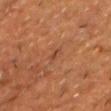{
  "biopsy_status": "not biopsied; imaged during a skin examination",
  "lesion_size": {
    "long_diameter_mm_approx": 2.5
  },
  "image": {
    "source": "total-body photography crop",
    "field_of_view_mm": 15
  },
  "site": "chest",
  "automated_metrics": {
    "lesion_detection_confidence_0_100": 100
  },
  "lighting": "cross-polarized",
  "patient": {
    "sex": "male",
    "age_approx": 60
  }
}